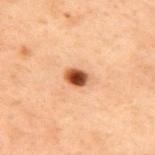Q: Was a biopsy performed?
A: imaged on a skin check; not biopsied
Q: What did automated image analysis measure?
A: a mean CIELAB color near L≈41 a*≈22 b*≈30 and a lesion-to-skin contrast of about 12.5 (normalized; higher = more distinct); a nevus-likeness score of about 100/100
Q: Lesion location?
A: the upper back
Q: What is the imaging modality?
A: total-body-photography crop, ~15 mm field of view
Q: Illumination type?
A: cross-polarized
Q: Patient demographics?
A: male, aged approximately 70
Q: How large is the lesion?
A: ≈3 mm